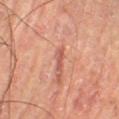notes: no biopsy performed (imaged during a skin exam); anatomic site: the right lower leg; lighting: cross-polarized illumination; diameter: about 2.5 mm; image source: ~15 mm tile from a whole-body skin photo; patient: male, roughly 55 years of age.